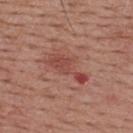Q: Is there a histopathology result?
A: imaged on a skin check; not biopsied
Q: Who is the patient?
A: male, approximately 55 years of age
Q: Lesion location?
A: the upper back
Q: How large is the lesion?
A: ~5.5 mm (longest diameter)
Q: What is the imaging modality?
A: ~15 mm crop, total-body skin-cancer survey
Q: What lighting was used for the tile?
A: white-light